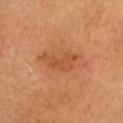Case summary:
* notes: total-body-photography surveillance lesion; no biopsy
* imaging modality: ~15 mm crop, total-body skin-cancer survey
* lesion size: ~4.5 mm (longest diameter)
* lighting: cross-polarized
* automated metrics: roughly 8 lightness units darker than nearby skin and a lesion-to-skin contrast of about 6 (normalized; higher = more distinct); peripheral color asymmetry of about 0.5; a nevus-likeness score of about 20/100 and lesion-presence confidence of about 100/100
* anatomic site: the head or neck
* patient: female, approximately 65 years of age